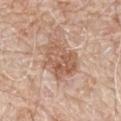This lesion was catalogued during total-body skin photography and was not selected for biopsy. Captured under white-light illumination. Automated image analysis of the tile measured a classifier nevus-likeness of about 0/100 and a lesion-detection confidence of about 100/100. The lesion is on the mid back. A roughly 15 mm field-of-view crop from a total-body skin photograph. A male patient about 80 years old.